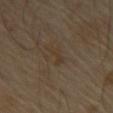  biopsy_status: not biopsied; imaged during a skin examination
  automated_metrics:
    eccentricity: 0.9
    shape_asymmetry: 0.4
    border_irregularity_0_10: 5.0
    peripheral_color_asymmetry: 0.0
    nevus_likeness_0_100: 0
    lesion_detection_confidence_0_100: 95
  image:
    source: total-body photography crop
    field_of_view_mm: 15
  lesion_size:
    long_diameter_mm_approx: 3.0
  lighting: cross-polarized
  patient:
    sex: male
    age_approx: 65